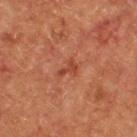Impression: This lesion was catalogued during total-body skin photography and was not selected for biopsy. Background: The lesion is on the upper back. Longest diameter approximately 2.5 mm. A male patient, in their mid-50s. This image is a 15 mm lesion crop taken from a total-body photograph.